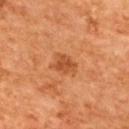The lesion was photographed on a routine skin check and not biopsied; there is no pathology result. A lesion tile, about 15 mm wide, cut from a 3D total-body photograph. Located on the upper back. Longest diameter approximately 3 mm. A female subject, aged 63–67. Captured under cross-polarized illumination.